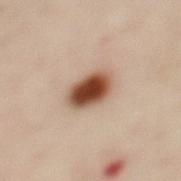Clinical impression:
Recorded during total-body skin imaging; not selected for excision or biopsy.
Clinical summary:
A region of skin cropped from a whole-body photographic capture, roughly 15 mm wide. A female subject, aged around 40. The tile uses cross-polarized illumination. The lesion's longest dimension is about 4.5 mm. The lesion-visualizer software estimated a lesion area of about 10 mm² and a shape-asymmetry score of about 0.1 (0 = symmetric). The software also gave about 19 CIELAB-L* units darker than the surrounding skin and a normalized lesion–skin contrast near 13.5. It also reported a peripheral color-asymmetry measure near 2. The analysis additionally found lesion-presence confidence of about 100/100. The lesion is on the upper back.